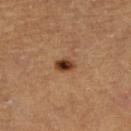<tbp_lesion>
<biopsy_status>not biopsied; imaged during a skin examination</biopsy_status>
<image>
  <source>total-body photography crop</source>
  <field_of_view_mm>15</field_of_view_mm>
</image>
<site>right lower leg</site>
<patient>
  <sex>male</sex>
  <age_approx>65</age_approx>
</patient>
</tbp_lesion>